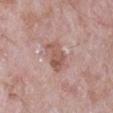Impression:
No biopsy was performed on this lesion — it was imaged during a full skin examination and was not determined to be concerning.
Acquisition and patient details:
The lesion-visualizer software estimated an outline eccentricity of about 0.8 (0 = round, 1 = elongated) and two-axis asymmetry of about 0.3. It also reported a border-irregularity rating of about 3/10, a color-variation rating of about 4/10, and a peripheral color-asymmetry measure near 1.5. And it measured a classifier nevus-likeness of about 5/100 and lesion-presence confidence of about 100/100. The lesion is located on the right upper arm. The lesion's longest dimension is about 4 mm. A lesion tile, about 15 mm wide, cut from a 3D total-body photograph. A male subject in their mid- to late 60s. Imaged with white-light lighting.